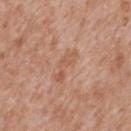The lesion was photographed on a routine skin check and not biopsied; there is no pathology result. A male subject, aged 63 to 67. From the back. Imaged with white-light lighting. Cropped from a whole-body photographic skin survey; the tile spans about 15 mm. Automated tile analysis of the lesion measured a border-irregularity index near 5.5/10, a color-variation rating of about 1/10, and a peripheral color-asymmetry measure near 0. The recorded lesion diameter is about 4 mm.